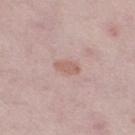No biopsy was performed on this lesion — it was imaged during a full skin examination and was not determined to be concerning.
A female subject approximately 45 years of age.
Located on the leg.
Cropped from a whole-body photographic skin survey; the tile spans about 15 mm.
Imaged with white-light lighting.
Longest diameter approximately 3 mm.